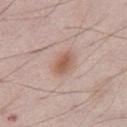workup: total-body-photography surveillance lesion; no biopsy
illumination: white-light illumination
lesion diameter: about 3 mm
subject: male, aged around 70
acquisition: total-body-photography crop, ~15 mm field of view
location: the abdomen
automated lesion analysis: an eccentricity of roughly 0.75 and a shape-asymmetry score of about 0.15 (0 = symmetric); an automated nevus-likeness rating near 90 out of 100 and lesion-presence confidence of about 100/100